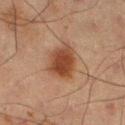Assessment: This lesion was catalogued during total-body skin photography and was not selected for biopsy. Clinical summary: The lesion is on the left thigh. A male subject, about 65 years old. A 15 mm crop from a total-body photograph taken for skin-cancer surveillance.